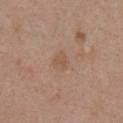The lesion was photographed on a routine skin check and not biopsied; there is no pathology result.